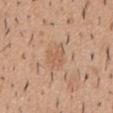workup: imaged on a skin check; not biopsied
body site: the mid back
size: ≈3.5 mm
subject: male, roughly 40 years of age
tile lighting: white-light
image source: 15 mm crop, total-body photography